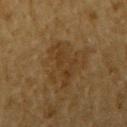Part of a total-body skin-imaging series; this lesion was reviewed on a skin check and was not flagged for biopsy. A male subject, about 85 years old or older. The lesion is located on the right upper arm. A lesion tile, about 15 mm wide, cut from a 3D total-body photograph.